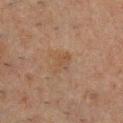Imaged during a routine full-body skin examination; the lesion was not biopsied and no histopathology is available. A male patient aged approximately 50. The lesion is located on the chest. A close-up tile cropped from a whole-body skin photograph, about 15 mm across.